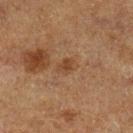This lesion was catalogued during total-body skin photography and was not selected for biopsy. A roughly 15 mm field-of-view crop from a total-body skin photograph. The lesion is located on the left lower leg. A male subject approximately 75 years of age. Imaged with cross-polarized lighting.